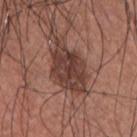biopsy status: total-body-photography surveillance lesion; no biopsy
lesion diameter: ≈6.5 mm
location: the chest
image: ~15 mm tile from a whole-body skin photo
lighting: white-light illumination
automated metrics: a lesion area of about 16 mm² and an eccentricity of roughly 0.8; a lesion color around L≈39 a*≈20 b*≈23 in CIELAB and about 12 CIELAB-L* units darker than the surrounding skin; a border-irregularity rating of about 3/10 and internal color variation of about 3.5 on a 0–10 scale
patient: male, aged approximately 35